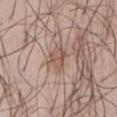{
  "biopsy_status": "not biopsied; imaged during a skin examination",
  "patient": {
    "sex": "male",
    "age_approx": 55
  },
  "lighting": "white-light",
  "image": {
    "source": "total-body photography crop",
    "field_of_view_mm": 15
  },
  "site": "abdomen",
  "lesion_size": {
    "long_diameter_mm_approx": 3.5
  },
  "automated_metrics": {
    "nevus_likeness_0_100": 0,
    "lesion_detection_confidence_0_100": 55
  }
}